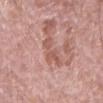workup — no biopsy performed (imaged during a skin exam)
site — the left forearm
illumination — white-light
patient — male, aged around 65
image — total-body-photography crop, ~15 mm field of view
lesion diameter — about 4 mm